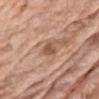Assessment: Recorded during total-body skin imaging; not selected for excision or biopsy. Context: Cropped from a whole-body photographic skin survey; the tile spans about 15 mm. A male subject, in their mid- to late 70s. On the front of the torso.